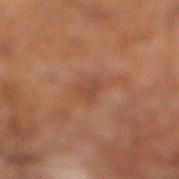Q: What is the imaging modality?
A: ~15 mm tile from a whole-body skin photo
Q: Lesion size?
A: ≈2.5 mm
Q: What did automated image analysis measure?
A: a lesion area of about 4 mm²; an average lesion color of about L≈36 a*≈19 b*≈27 (CIELAB), roughly 5 lightness units darker than nearby skin, and a normalized border contrast of about 5
Q: What are the patient's age and sex?
A: male, aged around 65
Q: Lesion location?
A: the right lower leg